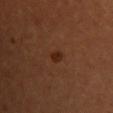Impression: This lesion was catalogued during total-body skin photography and was not selected for biopsy. Acquisition and patient details: The subject is a female roughly 65 years of age. The lesion is located on the upper back. This image is a 15 mm lesion crop taken from a total-body photograph.